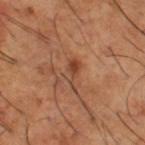Assessment: The lesion was photographed on a routine skin check and not biopsied; there is no pathology result. Image and clinical context: This is a cross-polarized tile. A male patient, roughly 65 years of age. A 15 mm close-up extracted from a 3D total-body photography capture. The total-body-photography lesion software estimated border irregularity of about 4.5 on a 0–10 scale, internal color variation of about 5 on a 0–10 scale, and radial color variation of about 1.5. The analysis additionally found a nevus-likeness score of about 0/100 and a detector confidence of about 100 out of 100 that the crop contains a lesion. On the right thigh.